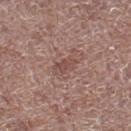{
  "site": "left lower leg",
  "image": {
    "source": "total-body photography crop",
    "field_of_view_mm": 15
  },
  "lesion_size": {
    "long_diameter_mm_approx": 3.0
  },
  "patient": {
    "sex": "male",
    "age_approx": 70
  }
}